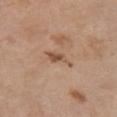workup = no biopsy performed (imaged during a skin exam); patient = female, aged approximately 55; site = the chest; imaging modality = total-body-photography crop, ~15 mm field of view; lighting = white-light illumination.